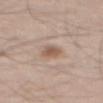{"biopsy_status": "not biopsied; imaged during a skin examination", "image": {"source": "total-body photography crop", "field_of_view_mm": 15}, "patient": {"sex": "male", "age_approx": 50}, "site": "back"}